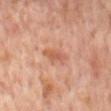workup — imaged on a skin check; not biopsied
patient — male, aged approximately 65
location — the back
acquisition — ~15 mm tile from a whole-body skin photo